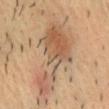Assessment:
Captured during whole-body skin photography for melanoma surveillance; the lesion was not biopsied.
Context:
A male subject aged approximately 40. Captured under cross-polarized illumination. Cropped from a whole-body photographic skin survey; the tile spans about 15 mm. Measured at roughly 10.5 mm in maximum diameter. Located on the front of the torso. Automated image analysis of the tile measured a border-irregularity index near 9/10, a within-lesion color-variation index near 5.5/10, and radial color variation of about 1.5. And it measured an automated nevus-likeness rating near 90 out of 100.